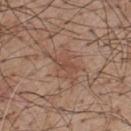Q: Was a biopsy performed?
A: catalogued during a skin exam; not biopsied
Q: How was this image acquired?
A: 15 mm crop, total-body photography
Q: Who is the patient?
A: male, in their mid-50s
Q: Lesion location?
A: the upper back